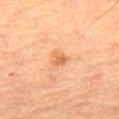workup: total-body-photography surveillance lesion; no biopsy
site: the right thigh
imaging modality: ~15 mm crop, total-body skin-cancer survey
TBP lesion metrics: a lesion color around L≈60 a*≈24 b*≈40 in CIELAB and roughly 9 lightness units darker than nearby skin; a classifier nevus-likeness of about 30/100 and lesion-presence confidence of about 100/100
patient: male, about 65 years old
lesion diameter: ~2 mm (longest diameter)